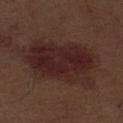image: ~15 mm crop, total-body skin-cancer survey; subject: male, about 70 years old; tile lighting: white-light; site: the abdomen.